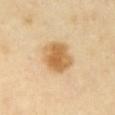Findings:
– workup: imaged on a skin check; not biopsied
– lighting: cross-polarized illumination
– location: the chest
– subject: female, aged approximately 60
– imaging modality: total-body-photography crop, ~15 mm field of view